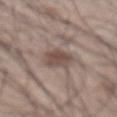Assessment:
The lesion was tiled from a total-body skin photograph and was not biopsied.
Background:
A male patient, aged around 45. A 15 mm crop from a total-body photograph taken for skin-cancer surveillance. From the front of the torso.